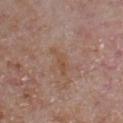Case summary:
- notes — total-body-photography surveillance lesion; no biopsy
- image — ~15 mm tile from a whole-body skin photo
- image-analysis metrics — about 5 CIELAB-L* units darker than the surrounding skin and a normalized border contrast of about 6
- tile lighting — white-light illumination
- patient — male, aged approximately 65
- location — the chest